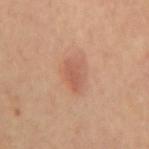Findings:
• biopsy status: total-body-photography surveillance lesion; no biopsy
• TBP lesion metrics: a mean CIELAB color near L≈58 a*≈25 b*≈31, a lesion–skin lightness drop of about 8, and a lesion-to-skin contrast of about 5.5 (normalized; higher = more distinct); border irregularity of about 2.5 on a 0–10 scale and a color-variation rating of about 2/10; a lesion-detection confidence of about 100/100
• image source: 15 mm crop, total-body photography
• location: the mid back
• tile lighting: cross-polarized illumination